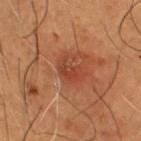{"biopsy_status": "not biopsied; imaged during a skin examination", "patient": {"sex": "male", "age_approx": 50}, "image": {"source": "total-body photography crop", "field_of_view_mm": 15}, "lighting": "cross-polarized", "site": "chest"}